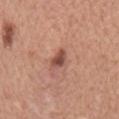This lesion was catalogued during total-body skin photography and was not selected for biopsy. A male patient roughly 65 years of age. A 15 mm close-up extracted from a 3D total-body photography capture. An algorithmic analysis of the crop reported a nevus-likeness score of about 85/100 and lesion-presence confidence of about 100/100. On the mid back. Imaged with white-light lighting.